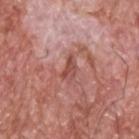Q: Was this lesion biopsied?
A: imaged on a skin check; not biopsied
Q: Who is the patient?
A: male, in their 60s
Q: Lesion size?
A: ≈3 mm
Q: Automated lesion metrics?
A: an area of roughly 3.5 mm² and a shape-asymmetry score of about 0.55 (0 = symmetric); an average lesion color of about L≈49 a*≈27 b*≈26 (CIELAB), roughly 10 lightness units darker than nearby skin, and a normalized border contrast of about 7; a classifier nevus-likeness of about 0/100
Q: How was the tile lit?
A: white-light illumination
Q: What is the anatomic site?
A: the upper back
Q: What kind of image is this?
A: total-body-photography crop, ~15 mm field of view